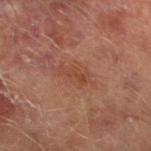The lesion was photographed on a routine skin check and not biopsied; there is no pathology result. A roughly 15 mm field-of-view crop from a total-body skin photograph. Captured under cross-polarized illumination. The lesion is on the left lower leg. Automated image analysis of the tile measured an average lesion color of about L≈44 a*≈24 b*≈30 (CIELAB), about 6 CIELAB-L* units darker than the surrounding skin, and a normalized border contrast of about 5.5. And it measured a border-irregularity rating of about 4.5/10, a within-lesion color-variation index near 2/10, and peripheral color asymmetry of about 0.5. It also reported a classifier nevus-likeness of about 0/100 and a detector confidence of about 100 out of 100 that the crop contains a lesion. The recorded lesion diameter is about 3.5 mm. The subject is a male aged around 65.